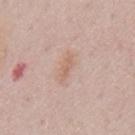location: the chest; image: ~15 mm tile from a whole-body skin photo; illumination: white-light illumination; subject: male, aged 48 to 52; lesion size: ≈3 mm.